A 15 mm close-up extracted from a 3D total-body photography capture. From the chest. A male subject aged 78 to 82. This is a white-light tile.
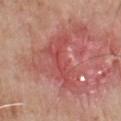Q: What is the histopathologic diagnosis?
A: a nodular basal cell carcinoma